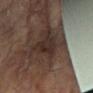Impression:
The lesion was photographed on a routine skin check and not biopsied; there is no pathology result.
Context:
A 15 mm close-up extracted from a 3D total-body photography capture. An algorithmic analysis of the crop reported roughly 7 lightness units darker than nearby skin and a lesion-to-skin contrast of about 8 (normalized; higher = more distinct). The software also gave a border-irregularity index near 3.5/10, a color-variation rating of about 2/10, and a peripheral color-asymmetry measure near 1. It also reported a nevus-likeness score of about 0/100 and lesion-presence confidence of about 60/100. A female patient in their 80s. On the left arm.